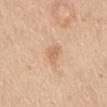image-analysis metrics: a lesion color around L≈66 a*≈19 b*≈35 in CIELAB and about 7 CIELAB-L* units darker than the surrounding skin; an automated nevus-likeness rating near 0 out of 100 and a lesion-detection confidence of about 100/100
lighting: white-light illumination
site: the back
patient: female, aged around 40
lesion diameter: ~2.5 mm (longest diameter)
image source: total-body-photography crop, ~15 mm field of view